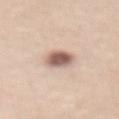lighting: white-light
patient:
  sex: female
  age_approx: 25
image:
  source: total-body photography crop
  field_of_view_mm: 15
lesion_size:
  long_diameter_mm_approx: 3.5
site: abdomen